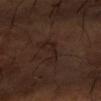{"biopsy_status": "not biopsied; imaged during a skin examination", "lesion_size": {"long_diameter_mm_approx": 4.5}, "image": {"source": "total-body photography crop", "field_of_view_mm": 15}, "lighting": "cross-polarized", "site": "left forearm", "patient": {"sex": "male", "age_approx": 65}, "automated_metrics": {"area_mm2_approx": 5.0, "eccentricity": 0.95, "shape_asymmetry": 0.65, "border_irregularity_0_10": 8.0, "color_variation_0_10": 1.5, "peripheral_color_asymmetry": 0.5, "nevus_likeness_0_100": 0, "lesion_detection_confidence_0_100": 95}}